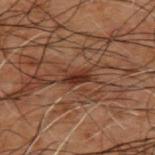workup: catalogued during a skin exam; not biopsied
subject: male, in their 50s
anatomic site: the upper back
imaging modality: ~15 mm crop, total-body skin-cancer survey
illumination: cross-polarized
image-analysis metrics: a lesion color around L≈23 a*≈18 b*≈22 in CIELAB, a lesion–skin lightness drop of about 9, and a lesion-to-skin contrast of about 10 (normalized; higher = more distinct); a border-irregularity rating of about 3/10 and a peripheral color-asymmetry measure near 0.5; a classifier nevus-likeness of about 15/100 and a detector confidence of about 85 out of 100 that the crop contains a lesion
size: ≈3 mm